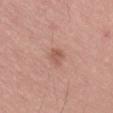Part of a total-body skin-imaging series; this lesion was reviewed on a skin check and was not flagged for biopsy. A region of skin cropped from a whole-body photographic capture, roughly 15 mm wide. Approximately 3 mm at its widest. The patient is a male approximately 55 years of age. The lesion is on the lower back. Captured under white-light illumination.